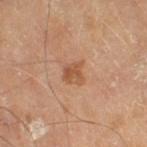| feature | finding |
|---|---|
| follow-up | catalogued during a skin exam; not biopsied |
| tile lighting | cross-polarized |
| size | ≈2.5 mm |
| automated lesion analysis | a within-lesion color-variation index near 3/10 and peripheral color asymmetry of about 1 |
| anatomic site | the right thigh |
| subject | male, approximately 65 years of age |
| imaging modality | ~15 mm crop, total-body skin-cancer survey |